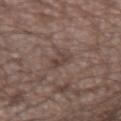  biopsy_status: not biopsied; imaged during a skin examination
  site: right forearm
  lesion_size:
    long_diameter_mm_approx: 2.5
  patient:
    sex: male
    age_approx: 65
  image:
    source: total-body photography crop
    field_of_view_mm: 15